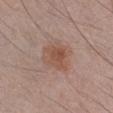Clinical impression: This lesion was catalogued during total-body skin photography and was not selected for biopsy. Clinical summary: A region of skin cropped from a whole-body photographic capture, roughly 15 mm wide. Located on the chest. The patient is a male aged 23–27.